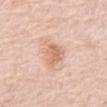This lesion was catalogued during total-body skin photography and was not selected for biopsy.
A roughly 15 mm field-of-view crop from a total-body skin photograph.
The patient is a male approximately 80 years of age.
The lesion is on the abdomen.
The recorded lesion diameter is about 4 mm.